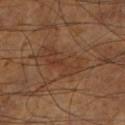Q: Was a biopsy performed?
A: catalogued during a skin exam; not biopsied